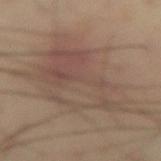Q: Was a biopsy performed?
A: total-body-photography surveillance lesion; no biopsy
Q: What is the imaging modality?
A: ~15 mm tile from a whole-body skin photo
Q: What is the anatomic site?
A: the leg
Q: What are the patient's age and sex?
A: male, in their mid- to late 50s